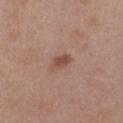No biopsy was performed on this lesion — it was imaged during a full skin examination and was not determined to be concerning. About 2.5 mm across. The total-body-photography lesion software estimated an outline eccentricity of about 0.75 (0 = round, 1 = elongated). The analysis additionally found an average lesion color of about L≈49 a*≈21 b*≈26 (CIELAB), roughly 9 lightness units darker than nearby skin, and a normalized border contrast of about 7. The tile uses white-light illumination. A lesion tile, about 15 mm wide, cut from a 3D total-body photograph. From the chest. A female subject, aged 38–42.